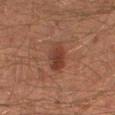| key | value |
|---|---|
| follow-up | catalogued during a skin exam; not biopsied |
| image | ~15 mm tile from a whole-body skin photo |
| subject | male, about 45 years old |
| lesion size | about 3.5 mm |
| site | the right lower leg |